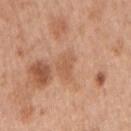| field | value |
|---|---|
| patient | female, aged 38 to 42 |
| diameter | about 3 mm |
| imaging modality | 15 mm crop, total-body photography |
| body site | the right upper arm |
| image-analysis metrics | an area of roughly 5.5 mm²; an average lesion color of about L≈58 a*≈22 b*≈34 (CIELAB), roughly 7 lightness units darker than nearby skin, and a lesion-to-skin contrast of about 5 (normalized; higher = more distinct); an automated nevus-likeness rating near 0 out of 100 and lesion-presence confidence of about 100/100 |
| tile lighting | white-light |